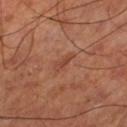Clinical impression:
The lesion was photographed on a routine skin check and not biopsied; there is no pathology result.
Image and clinical context:
Approximately 2.5 mm at its widest. A close-up tile cropped from a whole-body skin photograph, about 15 mm across. Captured under cross-polarized illumination. A male patient, aged 68 to 72. Located on the left thigh.